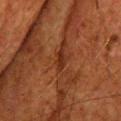follow-up = no biopsy performed (imaged during a skin exam) | lesion diameter = about 3 mm | image = ~15 mm tile from a whole-body skin photo | patient = male, in their mid-60s | anatomic site = the head or neck.